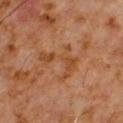<lesion>
  <biopsy_status>not biopsied; imaged during a skin examination</biopsy_status>
  <patient>
    <sex>male</sex>
    <age_approx>60</age_approx>
  </patient>
  <site>chest</site>
  <image>
    <source>total-body photography crop</source>
    <field_of_view_mm>15</field_of_view_mm>
  </image>
  <lesion_size>
    <long_diameter_mm_approx>4.5</long_diameter_mm_approx>
  </lesion_size>
  <lighting>cross-polarized</lighting>
</lesion>